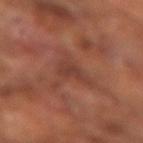Q: Was a biopsy performed?
A: catalogued during a skin exam; not biopsied
Q: How large is the lesion?
A: ~3 mm (longest diameter)
Q: Patient demographics?
A: male, in their mid-60s
Q: What kind of image is this?
A: ~15 mm crop, total-body skin-cancer survey
Q: What lighting was used for the tile?
A: cross-polarized
Q: Lesion location?
A: the left forearm